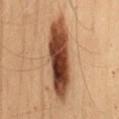• biopsy status — total-body-photography surveillance lesion; no biopsy
• patient — female, roughly 50 years of age
• lesion size — ≈9 mm
• acquisition — ~15 mm tile from a whole-body skin photo
• location — the mid back
• illumination — cross-polarized illumination